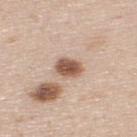The lesion was photographed on a routine skin check and not biopsied; there is no pathology result.
Longest diameter approximately 3 mm.
From the upper back.
Imaged with white-light lighting.
A 15 mm close-up tile from a total-body photography series done for melanoma screening.
A male patient, aged 43–47.